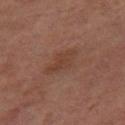biopsy status = catalogued during a skin exam; not biopsied | image source = ~15 mm tile from a whole-body skin photo | illumination = cross-polarized | anatomic site = the right thigh | subject = female, about 60 years old | diameter = ~4.5 mm (longest diameter).